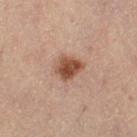| field | value |
|---|---|
| biopsy status | total-body-photography surveillance lesion; no biopsy |
| patient | female, aged 63–67 |
| location | the left thigh |
| image source | ~15 mm crop, total-body skin-cancer survey |
| tile lighting | cross-polarized |
| automated lesion analysis | a lesion area of about 7 mm² and a symmetry-axis asymmetry near 0.25; an average lesion color of about L≈49 a*≈22 b*≈30 (CIELAB), roughly 14 lightness units darker than nearby skin, and a normalized border contrast of about 10; border irregularity of about 2.5 on a 0–10 scale, a within-lesion color-variation index near 4.5/10, and peripheral color asymmetry of about 1.5 |